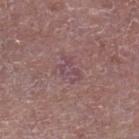| field | value |
|---|---|
| workup | total-body-photography surveillance lesion; no biopsy |
| subject | male, in their mid-60s |
| location | the left lower leg |
| automated metrics | an area of roughly 5.5 mm², a shape eccentricity near 0.75, and a symmetry-axis asymmetry near 0.35; a border-irregularity index near 4.5/10, a within-lesion color-variation index near 3/10, and peripheral color asymmetry of about 1; a lesion-detection confidence of about 95/100 |
| acquisition | total-body-photography crop, ~15 mm field of view |